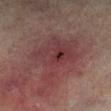No biopsy was performed on this lesion — it was imaged during a full skin examination and was not determined to be concerning.
Measured at roughly 7 mm in maximum diameter.
A male patient, about 75 years old.
This image is a 15 mm lesion crop taken from a total-body photograph.
This is a cross-polarized tile.
The lesion is located on the right lower leg.